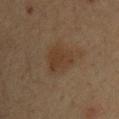<lesion>
<biopsy_status>not biopsied; imaged during a skin examination</biopsy_status>
<site>chest</site>
<patient>
  <sex>male</sex>
  <age_approx>35</age_approx>
</patient>
<lesion_size>
  <long_diameter_mm_approx>4.0</long_diameter_mm_approx>
</lesion_size>
<lighting>cross-polarized</lighting>
<image>
  <source>total-body photography crop</source>
  <field_of_view_mm>15</field_of_view_mm>
</image>
</lesion>